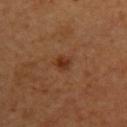{"biopsy_status": "not biopsied; imaged during a skin examination", "automated_metrics": {"cielab_L": 34, "cielab_a": 23, "cielab_b": 32, "vs_skin_darker_L": 8.0, "vs_skin_contrast_norm": 7.5, "border_irregularity_0_10": 2.0, "peripheral_color_asymmetry": 1.0, "nevus_likeness_0_100": 85}, "lighting": "cross-polarized", "image": {"source": "total-body photography crop", "field_of_view_mm": 15}, "site": "upper back", "lesion_size": {"long_diameter_mm_approx": 2.0}, "patient": {"sex": "female", "age_approx": 35}}